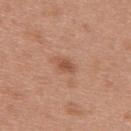The lesion was tiled from a total-body skin photograph and was not biopsied.
A region of skin cropped from a whole-body photographic capture, roughly 15 mm wide.
The recorded lesion diameter is about 2.5 mm.
A female patient roughly 40 years of age.
The lesion is located on the back.